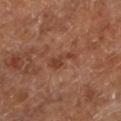biopsy status: catalogued during a skin exam; not biopsied | image: ~15 mm tile from a whole-body skin photo | anatomic site: the left lower leg | subject: in their mid-60s.